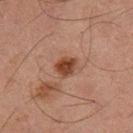Captured during whole-body skin photography for melanoma surveillance; the lesion was not biopsied. The tile uses cross-polarized illumination. Longest diameter approximately 2.5 mm. The subject is a male approximately 65 years of age. The total-body-photography lesion software estimated a color-variation rating of about 3/10 and peripheral color asymmetry of about 1. The analysis additionally found a lesion-detection confidence of about 100/100. A 15 mm close-up tile from a total-body photography series done for melanoma screening. From the right thigh.